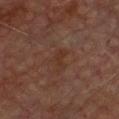Assessment: The lesion was tiled from a total-body skin photograph and was not biopsied. Acquisition and patient details: A male subject, aged around 75. Automated tile analysis of the lesion measured a within-lesion color-variation index near 1.5/10 and a peripheral color-asymmetry measure near 0.5. This is a cross-polarized tile. The lesion is located on the chest. A 15 mm close-up extracted from a 3D total-body photography capture.